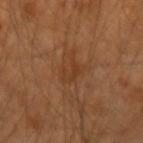Case summary:
– biopsy status: catalogued during a skin exam; not biopsied
– automated metrics: a lesion area of about 4 mm²; about 6 CIELAB-L* units darker than the surrounding skin; a border-irregularity rating of about 6.5/10, a within-lesion color-variation index near 1.5/10, and radial color variation of about 0.5
– illumination: cross-polarized illumination
– imaging modality: total-body-photography crop, ~15 mm field of view
– location: the arm
– subject: male, in their 50s
– lesion diameter: ~3.5 mm (longest diameter)